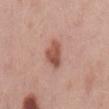| feature | finding |
|---|---|
| patient | male, aged 48–52 |
| image | ~15 mm crop, total-body skin-cancer survey |
| anatomic site | the mid back |